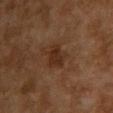Case summary:
- workup — catalogued during a skin exam; not biopsied
- illumination — cross-polarized illumination
- acquisition — total-body-photography crop, ~15 mm field of view
- size — ≈3 mm
- subject — female, about 60 years old
- anatomic site — the upper back